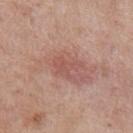| key | value |
|---|---|
| follow-up | catalogued during a skin exam; not biopsied |
| patient | male, roughly 55 years of age |
| acquisition | ~15 mm crop, total-body skin-cancer survey |
| size | about 3 mm |
| location | the left upper arm |
| TBP lesion metrics | a footprint of about 5 mm² and a symmetry-axis asymmetry near 0.5; a classifier nevus-likeness of about 0/100 and lesion-presence confidence of about 100/100 |